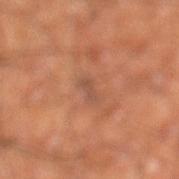• workup: imaged on a skin check; not biopsied
• patient: male, about 60 years old
• lighting: cross-polarized illumination
• automated metrics: a lesion color around L≈45 a*≈20 b*≈28 in CIELAB, about 6 CIELAB-L* units darker than the surrounding skin, and a lesion-to-skin contrast of about 5.5 (normalized; higher = more distinct); a nevus-likeness score of about 0/100 and a detector confidence of about 80 out of 100 that the crop contains a lesion
• acquisition: ~15 mm tile from a whole-body skin photo
• location: the left lower leg
• lesion size: ≈2.5 mm